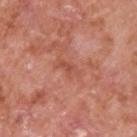Captured during whole-body skin photography for melanoma surveillance; the lesion was not biopsied. The lesion is on the upper back. A male patient roughly 65 years of age. Measured at roughly 3 mm in maximum diameter. The tile uses white-light illumination. A 15 mm crop from a total-body photograph taken for skin-cancer surveillance.